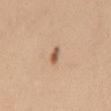This lesion was catalogued during total-body skin photography and was not selected for biopsy. The patient is a female about 30 years old. Measured at roughly 2.5 mm in maximum diameter. A lesion tile, about 15 mm wide, cut from a 3D total-body photograph. From the back. Automated image analysis of the tile measured a footprint of about 2.5 mm² and an outline eccentricity of about 0.9 (0 = round, 1 = elongated). And it measured a border-irregularity index near 3/10, internal color variation of about 0 on a 0–10 scale, and a peripheral color-asymmetry measure near 0. And it measured an automated nevus-likeness rating near 90 out of 100 and a detector confidence of about 100 out of 100 that the crop contains a lesion. Imaged with white-light lighting.